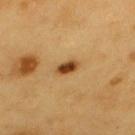{"biopsy_status": "not biopsied; imaged during a skin examination", "image": {"source": "total-body photography crop", "field_of_view_mm": 15}, "lighting": "cross-polarized", "site": "back", "lesion_size": {"long_diameter_mm_approx": 3.0}, "patient": {"sex": "male", "age_approx": 60}}